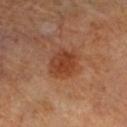<case>
  <biopsy_status>not biopsied; imaged during a skin examination</biopsy_status>
  <site>left lower leg</site>
  <lighting>cross-polarized</lighting>
  <patient>
    <age_approx>70</age_approx>
  </patient>
  <lesion_size>
    <long_diameter_mm_approx>4.0</long_diameter_mm_approx>
  </lesion_size>
  <image>
    <source>total-body photography crop</source>
    <field_of_view_mm>15</field_of_view_mm>
  </image>
</case>